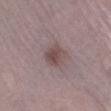workup: total-body-photography surveillance lesion; no biopsy
image source: ~15 mm tile from a whole-body skin photo
patient: female, aged approximately 70
location: the left lower leg